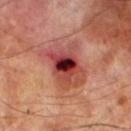The lesion was photographed on a routine skin check and not biopsied; there is no pathology result.
A close-up tile cropped from a whole-body skin photograph, about 15 mm across.
The subject is a male aged 68 to 72.
On the left lower leg.